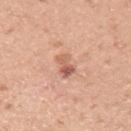{
  "biopsy_status": "not biopsied; imaged during a skin examination",
  "image": {
    "source": "total-body photography crop",
    "field_of_view_mm": 15
  },
  "site": "arm",
  "patient": {
    "sex": "male",
    "age_approx": 45
  },
  "lighting": "white-light",
  "automated_metrics": {
    "area_mm2_approx": 4.5,
    "eccentricity": 0.85,
    "shape_asymmetry": 0.25,
    "vs_skin_darker_L": 11.0,
    "lesion_detection_confidence_0_100": 100
  }
}